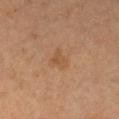{"biopsy_status": "not biopsied; imaged during a skin examination", "patient": {"sex": "female", "age_approx": 60}, "site": "chest", "lesion_size": {"long_diameter_mm_approx": 2.5}, "lighting": "cross-polarized", "image": {"source": "total-body photography crop", "field_of_view_mm": 15}}